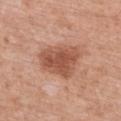Case summary:
* notes: total-body-photography surveillance lesion; no biopsy
* anatomic site: the chest
* image-analysis metrics: a footprint of about 16 mm² and a symmetry-axis asymmetry near 0.25; an automated nevus-likeness rating near 70 out of 100 and a lesion-detection confidence of about 100/100
* patient: female, in their mid- to late 70s
* illumination: white-light
* image source: ~15 mm tile from a whole-body skin photo
* size: ≈5 mm